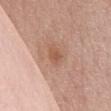| field | value |
|---|---|
| workup | total-body-photography surveillance lesion; no biopsy |
| body site | the chest |
| imaging modality | ~15 mm tile from a whole-body skin photo |
| patient | female, aged 63–67 |